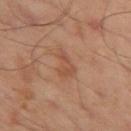A 15 mm close-up extracted from a 3D total-body photography capture. The tile uses cross-polarized illumination. Automated image analysis of the tile measured an area of roughly 5.5 mm² and a shape-asymmetry score of about 0.5 (0 = symmetric). The software also gave an average lesion color of about L≈50 a*≈22 b*≈31 (CIELAB) and about 7 CIELAB-L* units darker than the surrounding skin. And it measured a border-irregularity rating of about 5/10, internal color variation of about 2 on a 0–10 scale, and peripheral color asymmetry of about 0.5. It also reported a nevus-likeness score of about 0/100 and lesion-presence confidence of about 100/100. A male subject, in their mid-40s. Longest diameter approximately 4 mm. From the left thigh.